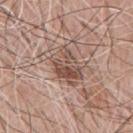Captured during whole-body skin photography for melanoma surveillance; the lesion was not biopsied. A male patient aged around 60. Cropped from a whole-body photographic skin survey; the tile spans about 15 mm. The lesion is on the chest.